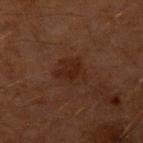{"lesion_size": {"long_diameter_mm_approx": 4.0}, "site": "left upper arm", "automated_metrics": {"area_mm2_approx": 9.0, "eccentricity": 0.55, "shape_asymmetry": 0.25, "border_irregularity_0_10": 3.0, "color_variation_0_10": 2.0, "peripheral_color_asymmetry": 1.0}, "lighting": "cross-polarized", "patient": {"sex": "male", "age_approx": 60}, "image": {"source": "total-body photography crop", "field_of_view_mm": 15}}